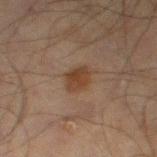Notes:
* site: the leg
* subject: male, aged approximately 70
* acquisition: 15 mm crop, total-body photography
* image-analysis metrics: a lesion color around L≈33 a*≈16 b*≈25 in CIELAB, about 7 CIELAB-L* units darker than the surrounding skin, and a lesion-to-skin contrast of about 8.5 (normalized; higher = more distinct); a border-irregularity rating of about 2.5/10, internal color variation of about 2 on a 0–10 scale, and radial color variation of about 0.5
* size: ≈3 mm
* tile lighting: cross-polarized